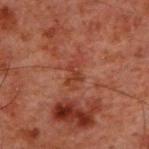biopsy status: imaged on a skin check; not biopsied
site: the upper back
diameter: about 2.5 mm
subject: male, aged approximately 60
acquisition: ~15 mm tile from a whole-body skin photo
tile lighting: cross-polarized